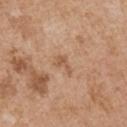{"biopsy_status": "not biopsied; imaged during a skin examination", "patient": {"sex": "male", "age_approx": 55}, "site": "right upper arm", "automated_metrics": {"area_mm2_approx": 3.0, "eccentricity": 0.85, "shape_asymmetry": 0.55, "cielab_L": 56, "cielab_a": 20, "cielab_b": 34, "vs_skin_darker_L": 8.0, "vs_skin_contrast_norm": 6.0}, "image": {"source": "total-body photography crop", "field_of_view_mm": 15}, "lighting": "white-light"}